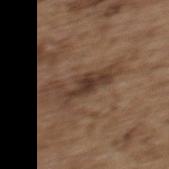image: 15 mm crop, total-body photography | anatomic site: the upper back | lighting: white-light | automated lesion analysis: a footprint of about 9 mm² and two-axis asymmetry of about 0.4; an average lesion color of about L≈37 a*≈16 b*≈25 (CIELAB), about 9 CIELAB-L* units darker than the surrounding skin, and a lesion-to-skin contrast of about 8 (normalized; higher = more distinct); a border-irregularity rating of about 7.5/10, internal color variation of about 3 on a 0–10 scale, and a peripheral color-asymmetry measure near 0.5; a classifier nevus-likeness of about 5/100 and a detector confidence of about 65 out of 100 that the crop contains a lesion | patient: female, roughly 65 years of age.